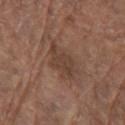Imaged during a routine full-body skin examination; the lesion was not biopsied and no histopathology is available.
A roughly 15 mm field-of-view crop from a total-body skin photograph.
Imaged with white-light lighting.
Located on the arm.
An algorithmic analysis of the crop reported a mean CIELAB color near L≈41 a*≈18 b*≈25, roughly 7 lightness units darker than nearby skin, and a normalized border contrast of about 6. It also reported a border-irregularity index near 4/10, a within-lesion color-variation index near 2.5/10, and peripheral color asymmetry of about 1. And it measured lesion-presence confidence of about 100/100.
The patient is a male aged 78–82.
Approximately 5 mm at its widest.